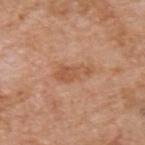Q: Was this lesion biopsied?
A: no biopsy performed (imaged during a skin exam)
Q: What are the patient's age and sex?
A: male, roughly 60 years of age
Q: How was this image acquired?
A: 15 mm crop, total-body photography
Q: What is the anatomic site?
A: the upper back